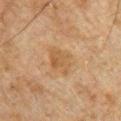No biopsy was performed on this lesion — it was imaged during a full skin examination and was not determined to be concerning. This is a cross-polarized tile. An algorithmic analysis of the crop reported an area of roughly 7 mm², a shape eccentricity near 0.65, and a symmetry-axis asymmetry near 0.25. It also reported a mean CIELAB color near L≈45 a*≈16 b*≈31 and a lesion-to-skin contrast of about 6 (normalized; higher = more distinct). The software also gave a border-irregularity rating of about 2.5/10 and peripheral color asymmetry of about 1. It also reported a nevus-likeness score of about 0/100 and lesion-presence confidence of about 100/100. A male subject aged approximately 50. Longest diameter approximately 3.5 mm. On the chest. A lesion tile, about 15 mm wide, cut from a 3D total-body photograph.